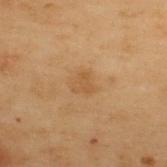This lesion was catalogued during total-body skin photography and was not selected for biopsy.
The lesion is located on the upper back.
A male patient about 55 years old.
A 15 mm close-up tile from a total-body photography series done for melanoma screening.